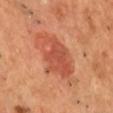biopsy status: total-body-photography surveillance lesion; no biopsy | patient: male, aged 48 to 52 | imaging modality: 15 mm crop, total-body photography | location: the chest.